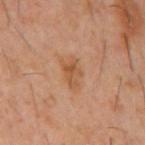Q: Is there a histopathology result?
A: total-body-photography surveillance lesion; no biopsy
Q: Who is the patient?
A: male, aged 58 to 62
Q: What kind of image is this?
A: ~15 mm tile from a whole-body skin photo
Q: Lesion location?
A: the mid back
Q: How was the tile lit?
A: cross-polarized illumination